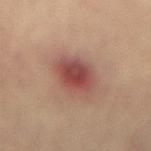Q: Was a biopsy performed?
A: total-body-photography surveillance lesion; no biopsy
Q: Automated lesion metrics?
A: a footprint of about 12 mm², an eccentricity of roughly 0.6, and two-axis asymmetry of about 0.1; a mean CIELAB color near L≈41 a*≈23 b*≈22, about 11 CIELAB-L* units darker than the surrounding skin, and a normalized lesion–skin contrast near 9
Q: How large is the lesion?
A: ~4.5 mm (longest diameter)
Q: Lesion location?
A: the lower back
Q: What is the imaging modality?
A: ~15 mm tile from a whole-body skin photo
Q: How was the tile lit?
A: cross-polarized
Q: What are the patient's age and sex?
A: male, aged around 65